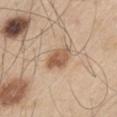The lesion was photographed on a routine skin check and not biopsied; there is no pathology result. This is a white-light tile. A male patient aged around 60. A 15 mm crop from a total-body photograph taken for skin-cancer surveillance. Located on the left thigh.